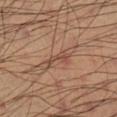The lesion was tiled from a total-body skin photograph and was not biopsied. A male patient, approximately 40 years of age. From the leg. A region of skin cropped from a whole-body photographic capture, roughly 15 mm wide.